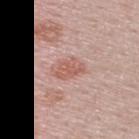Impression: The lesion was photographed on a routine skin check and not biopsied; there is no pathology result. Image and clinical context: Automated tile analysis of the lesion measured border irregularity of about 2.5 on a 0–10 scale and a color-variation rating of about 1.5/10. The analysis additionally found a nevus-likeness score of about 45/100 and a lesion-detection confidence of about 100/100. The lesion is located on the upper back. A roughly 15 mm field-of-view crop from a total-body skin photograph. A male patient roughly 60 years of age. Approximately 3.5 mm at its widest. Captured under white-light illumination.